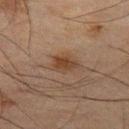The recorded lesion diameter is about 3 mm.
The lesion is located on the left thigh.
Imaged with cross-polarized lighting.
A region of skin cropped from a whole-body photographic capture, roughly 15 mm wide.
A male subject, in their mid- to late 60s.